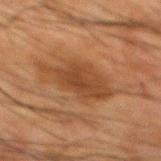follow-up = catalogued during a skin exam; not biopsied
size = about 6.5 mm
image source = 15 mm crop, total-body photography
patient = male, roughly 65 years of age
location = the mid back